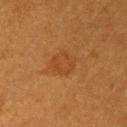No biopsy was performed on this lesion — it was imaged during a full skin examination and was not determined to be concerning. A female subject, aged 53 to 57. The lesion is on the chest. This is a cross-polarized tile. Longest diameter approximately 3 mm. A lesion tile, about 15 mm wide, cut from a 3D total-body photograph.